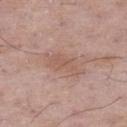biopsy_status: not biopsied; imaged during a skin examination
site: right thigh
lesion_size:
  long_diameter_mm_approx: 5.0
patient:
  sex: male
  age_approx: 50
image:
  source: total-body photography crop
  field_of_view_mm: 15
lighting: white-light